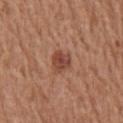| feature | finding |
|---|---|
| workup | catalogued during a skin exam; not biopsied |
| subject | male, aged approximately 65 |
| size | ~3 mm (longest diameter) |
| acquisition | ~15 mm crop, total-body skin-cancer survey |
| TBP lesion metrics | a mean CIELAB color near L≈45 a*≈25 b*≈29 and roughly 11 lightness units darker than nearby skin; border irregularity of about 2.5 on a 0–10 scale, a within-lesion color-variation index near 4/10, and radial color variation of about 1.5; a classifier nevus-likeness of about 80/100 |
| anatomic site | the chest |
| lighting | white-light illumination |